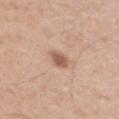Context: The lesion is located on the right upper arm. The patient is a male aged 33–37. A lesion tile, about 15 mm wide, cut from a 3D total-body photograph.